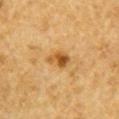Part of a total-body skin-imaging series; this lesion was reviewed on a skin check and was not flagged for biopsy. On the right upper arm. A 15 mm close-up tile from a total-body photography series done for melanoma screening. A male subject, aged approximately 85 or older.